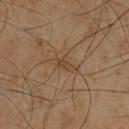On the chest. The tile uses cross-polarized illumination. A male patient roughly 60 years of age. The lesion-visualizer software estimated an area of roughly 3.5 mm², a shape eccentricity near 0.8, and a shape-asymmetry score of about 0.3 (0 = symmetric). The software also gave a lesion color around L≈39 a*≈14 b*≈29 in CIELAB, about 5 CIELAB-L* units darker than the surrounding skin, and a normalized border contrast of about 5.5. And it measured border irregularity of about 3.5 on a 0–10 scale and a within-lesion color-variation index near 2.5/10. This image is a 15 mm lesion crop taken from a total-body photograph. The recorded lesion diameter is about 3 mm.